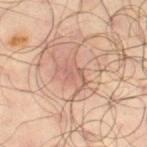Assessment:
The lesion was tiled from a total-body skin photograph and was not biopsied.
Acquisition and patient details:
Captured under cross-polarized illumination. A male subject about 65 years old. Automated tile analysis of the lesion measured a border-irregularity rating of about 8.5/10. This image is a 15 mm lesion crop taken from a total-body photograph. The lesion is located on the left thigh.